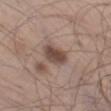Q: Is there a histopathology result?
A: total-body-photography surveillance lesion; no biopsy
Q: What did automated image analysis measure?
A: a footprint of about 6.5 mm² and a shape eccentricity near 0.8; a lesion color around L≈46 a*≈16 b*≈23 in CIELAB, roughly 13 lightness units darker than nearby skin, and a normalized border contrast of about 9.5; an automated nevus-likeness rating near 70 out of 100 and a lesion-detection confidence of about 100/100
Q: How was this image acquired?
A: total-body-photography crop, ~15 mm field of view
Q: Lesion location?
A: the left thigh
Q: What is the lesion's diameter?
A: ~3.5 mm (longest diameter)
Q: Patient demographics?
A: male, aged around 60